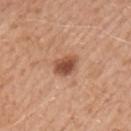No biopsy was performed on this lesion — it was imaged during a full skin examination and was not determined to be concerning.
A 15 mm crop from a total-body photograph taken for skin-cancer surveillance.
Located on the arm.
The subject is a male aged approximately 50.